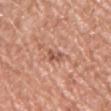Impression:
This lesion was catalogued during total-body skin photography and was not selected for biopsy.
Background:
The lesion is located on the chest. The recorded lesion diameter is about 3 mm. A male subject aged approximately 55. A close-up tile cropped from a whole-body skin photograph, about 15 mm across.